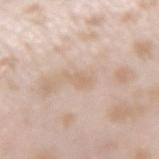The lesion was photographed on a routine skin check and not biopsied; there is no pathology result. About 2.5 mm across. On the right forearm. Automated tile analysis of the lesion measured a lesion area of about 3 mm², a shape eccentricity near 0.85, and a shape-asymmetry score of about 0.35 (0 = symmetric). It also reported an average lesion color of about L≈67 a*≈15 b*≈29 (CIELAB) and a lesion–skin lightness drop of about 6. And it measured a border-irregularity index near 3.5/10. Cropped from a whole-body photographic skin survey; the tile spans about 15 mm. A female subject about 25 years old.